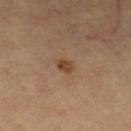Assessment:
Part of a total-body skin-imaging series; this lesion was reviewed on a skin check and was not flagged for biopsy.
Acquisition and patient details:
The lesion is located on the left thigh. The total-body-photography lesion software estimated a mean CIELAB color near L≈45 a*≈19 b*≈33, a lesion–skin lightness drop of about 9, and a normalized border contrast of about 8. This is a cross-polarized tile. A 15 mm crop from a total-body photograph taken for skin-cancer surveillance. Longest diameter approximately 2.5 mm. The patient is a female aged 68–72.